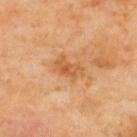biopsy status = no biopsy performed (imaged during a skin exam); image source = 15 mm crop, total-body photography; subject = male, roughly 70 years of age; location = the upper back.